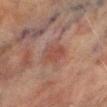<tbp_lesion>
  <biopsy_status>not biopsied; imaged during a skin examination</biopsy_status>
  <patient>
    <sex>male</sex>
    <age_approx>70</age_approx>
  </patient>
  <lighting>cross-polarized</lighting>
  <site>right lower leg</site>
  <lesion_size>
    <long_diameter_mm_approx>3.5</long_diameter_mm_approx>
  </lesion_size>
  <image>
    <source>total-body photography crop</source>
    <field_of_view_mm>15</field_of_view_mm>
  </image>
</tbp_lesion>